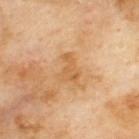Findings:
– automated metrics · a border-irregularity rating of about 6/10, internal color variation of about 2 on a 0–10 scale, and radial color variation of about 0.5
– lesion size · ~3.5 mm (longest diameter)
– anatomic site · the upper back
– imaging modality · ~15 mm tile from a whole-body skin photo
– subject · male, approximately 70 years of age
– lighting · cross-polarized